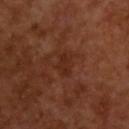Assessment: Part of a total-body skin-imaging series; this lesion was reviewed on a skin check and was not flagged for biopsy. Context: A lesion tile, about 15 mm wide, cut from a 3D total-body photograph. A male subject, approximately 65 years of age. Imaged with cross-polarized lighting. The lesion's longest dimension is about 2.5 mm.